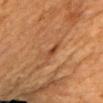notes=total-body-photography surveillance lesion; no biopsy
lesion size=about 3 mm
site=the arm
subject=female, aged approximately 55
tile lighting=cross-polarized illumination
image=~15 mm tile from a whole-body skin photo